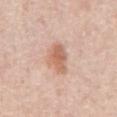{"lesion_size": {"long_diameter_mm_approx": 4.0}, "image": {"source": "total-body photography crop", "field_of_view_mm": 15}, "lighting": "white-light", "patient": {"sex": "male", "age_approx": 65}, "site": "abdomen"}